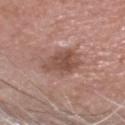Imaged during a routine full-body skin examination; the lesion was not biopsied and no histopathology is available.
The lesion's longest dimension is about 4.5 mm.
Automated image analysis of the tile measured a lesion color around L≈49 a*≈21 b*≈26 in CIELAB, about 11 CIELAB-L* units darker than the surrounding skin, and a normalized lesion–skin contrast near 7.5. And it measured a border-irregularity index near 3.5/10, a color-variation rating of about 4/10, and a peripheral color-asymmetry measure near 1.5. The software also gave lesion-presence confidence of about 100/100.
Located on the head or neck.
Captured under white-light illumination.
A 15 mm close-up extracted from a 3D total-body photography capture.
The patient is a male in their mid-60s.